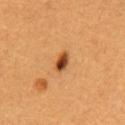  biopsy_status: not biopsied; imaged during a skin examination
  image:
    source: total-body photography crop
    field_of_view_mm: 15
  site: chest
  patient:
    sex: male
    age_approx: 60
  lesion_size:
    long_diameter_mm_approx: 2.5
  lighting: cross-polarized
  automated_metrics:
    area_mm2_approx: 3.5
    eccentricity: 0.85
    cielab_L: 43
    cielab_a: 25
    cielab_b: 38
    vs_skin_darker_L: 17.0